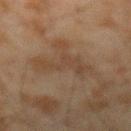This lesion was catalogued during total-body skin photography and was not selected for biopsy. The patient is a male in their mid-40s. Cropped from a total-body skin-imaging series; the visible field is about 15 mm. On the arm. The lesion-visualizer software estimated a footprint of about 7.5 mm², a shape eccentricity near 0.9, and a shape-asymmetry score of about 0.6 (0 = symmetric). It also reported border irregularity of about 9.5 on a 0–10 scale, internal color variation of about 1 on a 0–10 scale, and radial color variation of about 0.